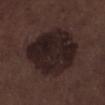The lesion was photographed on a routine skin check and not biopsied; there is no pathology result.
On the left lower leg.
The recorded lesion diameter is about 7 mm.
The patient is a male in their 70s.
A close-up tile cropped from a whole-body skin photograph, about 15 mm across.
Captured under white-light illumination.